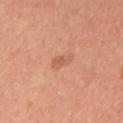Recorded during total-body skin imaging; not selected for excision or biopsy.
Cropped from a whole-body photographic skin survey; the tile spans about 15 mm.
A female patient about 30 years old.
Approximately 3 mm at its widest.
The lesion is located on the left upper arm.
The tile uses white-light illumination.